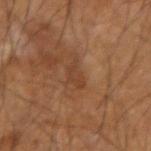notes = imaged on a skin check; not biopsied
subject = male, about 55 years old
illumination = cross-polarized
diameter = about 3 mm
image source = ~15 mm crop, total-body skin-cancer survey
body site = the arm